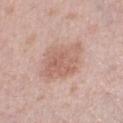Background:
Cropped from a total-body skin-imaging series; the visible field is about 15 mm. The patient is a female in their 60s. The total-body-photography lesion software estimated a lesion area of about 17 mm², an outline eccentricity of about 0.35 (0 = round, 1 = elongated), and a symmetry-axis asymmetry near 0.15. The analysis additionally found a lesion-to-skin contrast of about 6 (normalized; higher = more distinct). From the right thigh. Imaged with white-light lighting. Measured at roughly 4.5 mm in maximum diameter.